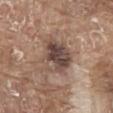location — the front of the torso
size — ~5 mm (longest diameter)
acquisition — ~15 mm crop, total-body skin-cancer survey
image-analysis metrics — a border-irregularity rating of about 2/10, a color-variation rating of about 8.5/10, and peripheral color asymmetry of about 3; a nevus-likeness score of about 20/100 and a detector confidence of about 100 out of 100 that the crop contains a lesion
tile lighting — white-light illumination
patient — male, roughly 80 years of age